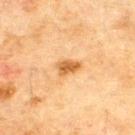follow-up: imaged on a skin check; not biopsied.